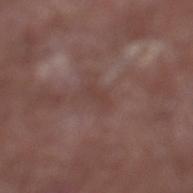Recorded during total-body skin imaging; not selected for excision or biopsy.
Located on the left lower leg.
A male subject roughly 65 years of age.
Cropped from a total-body skin-imaging series; the visible field is about 15 mm.